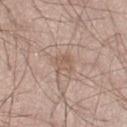Findings:
• notes: no biopsy performed (imaged during a skin exam)
• subject: male, roughly 30 years of age
• image source: ~15 mm crop, total-body skin-cancer survey
• anatomic site: the right thigh